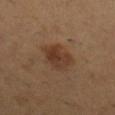The lesion was tiled from a total-body skin photograph and was not biopsied. A close-up tile cropped from a whole-body skin photograph, about 15 mm across. Automated image analysis of the tile measured a lesion color around L≈37 a*≈18 b*≈28 in CIELAB, about 8 CIELAB-L* units darker than the surrounding skin, and a normalized border contrast of about 7.5. The analysis additionally found a classifier nevus-likeness of about 95/100 and a lesion-detection confidence of about 100/100. Imaged with cross-polarized lighting. A female patient in their mid- to late 50s. Located on the right lower leg. Approximately 4 mm at its widest.